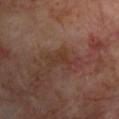Q: Is there a histopathology result?
A: imaged on a skin check; not biopsied
Q: What are the patient's age and sex?
A: male, approximately 70 years of age
Q: What is the lesion's diameter?
A: ≈2.5 mm
Q: What is the imaging modality?
A: ~15 mm crop, total-body skin-cancer survey
Q: Where on the body is the lesion?
A: the front of the torso